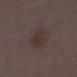Q: Was a biopsy performed?
A: no biopsy performed (imaged during a skin exam)
Q: Illumination type?
A: white-light
Q: What is the anatomic site?
A: the left lower leg
Q: What is the imaging modality?
A: total-body-photography crop, ~15 mm field of view
Q: How large is the lesion?
A: ~3 mm (longest diameter)
Q: Patient demographics?
A: female, in their 30s